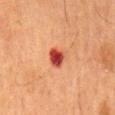follow-up: no biopsy performed (imaged during a skin exam) | patient: male, aged 63–67 | site: the mid back | lesion diameter: ~2.5 mm (longest diameter) | imaging modality: 15 mm crop, total-body photography.